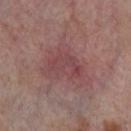notes: no biopsy performed (imaged during a skin exam); lighting: cross-polarized illumination; anatomic site: the left thigh; image: total-body-photography crop, ~15 mm field of view; subject: female, aged approximately 55.